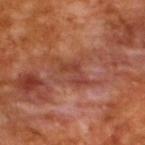Q: Was this lesion biopsied?
A: catalogued during a skin exam; not biopsied
Q: What kind of image is this?
A: ~15 mm crop, total-body skin-cancer survey
Q: How large is the lesion?
A: about 4.5 mm
Q: What did automated image analysis measure?
A: an area of roughly 5.5 mm², an eccentricity of roughly 0.85, and a shape-asymmetry score of about 0.55 (0 = symmetric); a border-irregularity index near 7/10, a within-lesion color-variation index near 2/10, and radial color variation of about 0; a nevus-likeness score of about 0/100 and a lesion-detection confidence of about 80/100
Q: What are the patient's age and sex?
A: male, aged 63–67